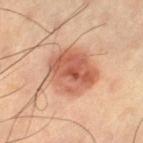Case summary:
* biopsy status — catalogued during a skin exam; not biopsied
* lighting — cross-polarized illumination
* image-analysis metrics — a mean CIELAB color near L≈58 a*≈26 b*≈33, about 13 CIELAB-L* units darker than the surrounding skin, and a normalized lesion–skin contrast near 8.5
* location — the left thigh
* subject — male, aged approximately 60
* image source — 15 mm crop, total-body photography
* diameter — ≈5.5 mm